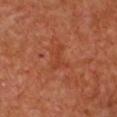Clinical impression:
This lesion was catalogued during total-body skin photography and was not selected for biopsy.
Context:
A female subject, approximately 55 years of age. Measured at roughly 3 mm in maximum diameter. Cropped from a whole-body photographic skin survey; the tile spans about 15 mm. The lesion is on the front of the torso.